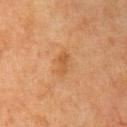biopsy status: imaged on a skin check; not biopsied | TBP lesion metrics: a footprint of about 3 mm² | subject: male, aged 78–82 | site: the chest | lighting: cross-polarized | imaging modality: ~15 mm tile from a whole-body skin photo.